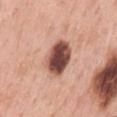A male subject aged around 60.
Captured under white-light illumination.
On the mid back.
Automated image analysis of the tile measured a lesion area of about 12 mm², an outline eccentricity of about 0.75 (0 = round, 1 = elongated), and a symmetry-axis asymmetry near 0.1. It also reported a mean CIELAB color near L≈48 a*≈24 b*≈26, about 22 CIELAB-L* units darker than the surrounding skin, and a normalized lesion–skin contrast near 14. The analysis additionally found a border-irregularity index near 1/10 and a peripheral color-asymmetry measure near 2.
About 4.5 mm across.
A lesion tile, about 15 mm wide, cut from a 3D total-body photograph.